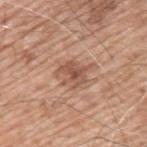On the upper back.
A lesion tile, about 15 mm wide, cut from a 3D total-body photograph.
The patient is a male aged around 60.